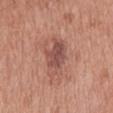| field | value |
|---|---|
| follow-up | catalogued during a skin exam; not biopsied |
| tile lighting | white-light illumination |
| image-analysis metrics | a lesion area of about 11 mm² and two-axis asymmetry of about 0.25; an automated nevus-likeness rating near 10 out of 100 and lesion-presence confidence of about 100/100 |
| anatomic site | the back |
| imaging modality | total-body-photography crop, ~15 mm field of view |
| patient | male, roughly 70 years of age |
| diameter | ~6 mm (longest diameter) |